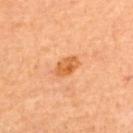{
  "biopsy_status": "not biopsied; imaged during a skin examination",
  "site": "upper back",
  "patient": {
    "sex": "female",
    "age_approx": 65
  },
  "lesion_size": {
    "long_diameter_mm_approx": 3.0
  },
  "automated_metrics": {
    "area_mm2_approx": 5.0,
    "eccentricity": 0.8,
    "shape_asymmetry": 0.25,
    "cielab_L": 59,
    "cielab_a": 27,
    "cielab_b": 44,
    "vs_skin_darker_L": 10.0,
    "vs_skin_contrast_norm": 8.0,
    "border_irregularity_0_10": 2.0,
    "color_variation_0_10": 3.5,
    "peripheral_color_asymmetry": 1.0,
    "nevus_likeness_0_100": 80,
    "lesion_detection_confidence_0_100": 100
  },
  "image": {
    "source": "total-body photography crop",
    "field_of_view_mm": 15
  }
}